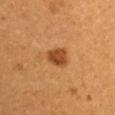illumination=cross-polarized illumination | subject=female, about 30 years old | body site=the left arm | image=total-body-photography crop, ~15 mm field of view.